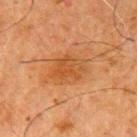The lesion was tiled from a total-body skin photograph and was not biopsied.